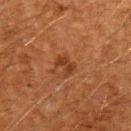| field | value |
|---|---|
| biopsy status | total-body-photography surveillance lesion; no biopsy |
| lesion size | about 2.5 mm |
| lighting | cross-polarized |
| image-analysis metrics | a lesion color around L≈30 a*≈21 b*≈29 in CIELAB and a lesion–skin lightness drop of about 7; a border-irregularity index near 4.5/10, internal color variation of about 1.5 on a 0–10 scale, and radial color variation of about 0.5; an automated nevus-likeness rating near 15 out of 100 |
| subject | male, about 60 years old |
| anatomic site | the left upper arm |
| imaging modality | total-body-photography crop, ~15 mm field of view |